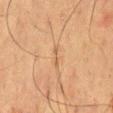Clinical impression: Part of a total-body skin-imaging series; this lesion was reviewed on a skin check and was not flagged for biopsy. Image and clinical context: Measured at roughly 3 mm in maximum diameter. The lesion is on the back. A roughly 15 mm field-of-view crop from a total-body skin photograph. A male subject about 60 years old.